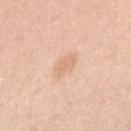This lesion was catalogued during total-body skin photography and was not selected for biopsy. On the right upper arm. The subject is a female about 40 years old. The lesion's longest dimension is about 3 mm. Captured under white-light illumination. Cropped from a whole-body photographic skin survey; the tile spans about 15 mm.